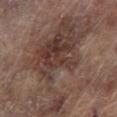The lesion was tiled from a total-body skin photograph and was not biopsied. This image is a 15 mm lesion crop taken from a total-body photograph. The lesion is on the left lower leg. About 13.5 mm across. A male patient approximately 65 years of age. This is a cross-polarized tile.